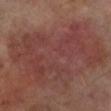Case summary:
- notes · imaged on a skin check; not biopsied
- TBP lesion metrics · an area of roughly 65 mm², an eccentricity of roughly 0.8, and a symmetry-axis asymmetry near 0.45; internal color variation of about 4 on a 0–10 scale and peripheral color asymmetry of about 1.5
- lesion diameter · ≈12.5 mm
- illumination · cross-polarized
- body site · the left lower leg
- patient · male, aged 68–72
- image · total-body-photography crop, ~15 mm field of view